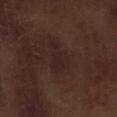No biopsy was performed on this lesion — it was imaged during a full skin examination and was not determined to be concerning. A region of skin cropped from a whole-body photographic capture, roughly 15 mm wide. A male subject aged 68 to 72. On the right lower leg.